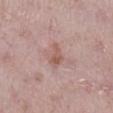follow-up=no biopsy performed (imaged during a skin exam) | patient=female, aged around 40 | automated lesion analysis=border irregularity of about 4.5 on a 0–10 scale; an automated nevus-likeness rating near 5 out of 100 and a lesion-detection confidence of about 100/100 | location=the leg | tile lighting=white-light illumination | size=≈3 mm | image source=total-body-photography crop, ~15 mm field of view.